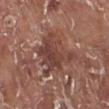notes: imaged on a skin check; not biopsied
size: ~6 mm (longest diameter)
image: 15 mm crop, total-body photography
location: the leg
patient: male, aged approximately 75
automated lesion analysis: a within-lesion color-variation index near 5/10 and a peripheral color-asymmetry measure near 1.5